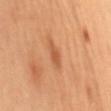Clinical impression:
Captured during whole-body skin photography for melanoma surveillance; the lesion was not biopsied.
Clinical summary:
About 3.5 mm across. A roughly 15 mm field-of-view crop from a total-body skin photograph. A female patient aged around 60. This is a cross-polarized tile. On the mid back. An algorithmic analysis of the crop reported a footprint of about 3 mm², an eccentricity of roughly 0.95, and a shape-asymmetry score of about 0.35 (0 = symmetric). It also reported an average lesion color of about L≈58 a*≈28 b*≈41 (CIELAB), roughly 10 lightness units darker than nearby skin, and a normalized lesion–skin contrast near 6.5.